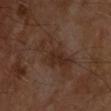{"biopsy_status": "not biopsied; imaged during a skin examination", "image": {"source": "total-body photography crop", "field_of_view_mm": 15}, "patient": {"sex": "male", "age_approx": 55}, "lesion_size": {"long_diameter_mm_approx": 6.0}, "site": "upper back", "lighting": "cross-polarized"}